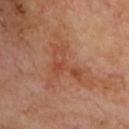Q: How large is the lesion?
A: about 8 mm
Q: How was the tile lit?
A: cross-polarized illumination
Q: What are the patient's age and sex?
A: male, aged around 70
Q: How was this image acquired?
A: ~15 mm tile from a whole-body skin photo
Q: Where on the body is the lesion?
A: the chest